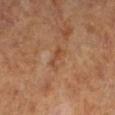workup = catalogued during a skin exam; not biopsied
diameter = about 3 mm
patient = female, aged 63 to 67
anatomic site = the leg
acquisition = ~15 mm tile from a whole-body skin photo
automated metrics = a classifier nevus-likeness of about 0/100 and a detector confidence of about 100 out of 100 that the crop contains a lesion
illumination = cross-polarized